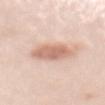Impression:
This lesion was catalogued during total-body skin photography and was not selected for biopsy.
Image and clinical context:
The tile uses white-light illumination. Cropped from a whole-body photographic skin survey; the tile spans about 15 mm. A female subject roughly 50 years of age. The lesion's longest dimension is about 5 mm. The lesion is located on the mid back.